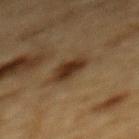biopsy status: total-body-photography surveillance lesion; no biopsy | diameter: about 3.5 mm | acquisition: total-body-photography crop, ~15 mm field of view | location: the mid back | subject: male, in their mid- to late 80s or older | tile lighting: cross-polarized.